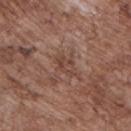On the chest. The patient is a male aged 68 to 72. This image is a 15 mm lesion crop taken from a total-body photograph.